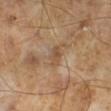follow-up — total-body-photography surveillance lesion; no biopsy | patient — male, in their mid- to late 60s | imaging modality — total-body-photography crop, ~15 mm field of view | lesion diameter — ≈2.5 mm.